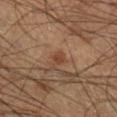Imaged during a routine full-body skin examination; the lesion was not biopsied and no histopathology is available.
From the right lower leg.
The total-body-photography lesion software estimated a shape eccentricity near 0.65 and a symmetry-axis asymmetry near 0.35. It also reported a border-irregularity rating of about 3/10, a within-lesion color-variation index near 0/10, and radial color variation of about 0.
A 15 mm crop from a total-body photograph taken for skin-cancer surveillance.
A male patient, approximately 60 years of age.
Measured at roughly 1.5 mm in maximum diameter.